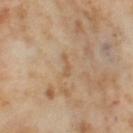workup: imaged on a skin check; not biopsied
acquisition: total-body-photography crop, ~15 mm field of view
tile lighting: cross-polarized
automated lesion analysis: an area of roughly 2 mm² and a shape-asymmetry score of about 0.4 (0 = symmetric); a lesion color around L≈58 a*≈16 b*≈34 in CIELAB and roughly 7 lightness units darker than nearby skin; a nevus-likeness score of about 0/100 and a lesion-detection confidence of about 100/100
lesion diameter: ~2.5 mm (longest diameter)
site: the left thigh
subject: female, in their mid-50s